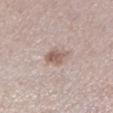<case>
<biopsy_status>not biopsied; imaged during a skin examination</biopsy_status>
<site>left lower leg</site>
<patient>
  <sex>female</sex>
  <age_approx>40</age_approx>
</patient>
<image>
  <source>total-body photography crop</source>
  <field_of_view_mm>15</field_of_view_mm>
</image>
</case>